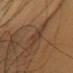This lesion was catalogued during total-body skin photography and was not selected for biopsy.
From the chest.
Imaged with cross-polarized lighting.
About 3.5 mm across.
A female patient, approximately 70 years of age.
Cropped from a whole-body photographic skin survey; the tile spans about 15 mm.
The lesion-visualizer software estimated a lesion color around L≈40 a*≈17 b*≈30 in CIELAB, about 6 CIELAB-L* units darker than the surrounding skin, and a lesion-to-skin contrast of about 5 (normalized; higher = more distinct). And it measured border irregularity of about 3 on a 0–10 scale, a color-variation rating of about 1/10, and a peripheral color-asymmetry measure near 0.5. It also reported a lesion-detection confidence of about 55/100.